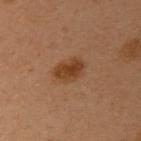notes = total-body-photography surveillance lesion; no biopsy
image source = ~15 mm crop, total-body skin-cancer survey
subject = female, aged approximately 50
location = the chest
illumination = cross-polarized illumination
lesion size = ~3.5 mm (longest diameter)
automated metrics = border irregularity of about 2.5 on a 0–10 scale, internal color variation of about 2.5 on a 0–10 scale, and a peripheral color-asymmetry measure near 0.5; a classifier nevus-likeness of about 100/100 and a detector confidence of about 100 out of 100 that the crop contains a lesion